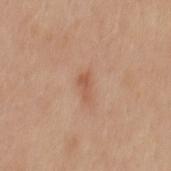Impression: This lesion was catalogued during total-body skin photography and was not selected for biopsy. Image and clinical context: Captured under white-light illumination. A region of skin cropped from a whole-body photographic capture, roughly 15 mm wide. Located on the back. A male subject about 30 years old. About 2.5 mm across.